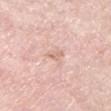Clinical summary: The tile uses white-light illumination. This image is a 15 mm lesion crop taken from a total-body photograph. Automated image analysis of the tile measured a lesion area of about 2.5 mm² and a symmetry-axis asymmetry near 0.4. The subject is a female aged 68 to 72. From the leg.